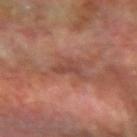The lesion is on the left forearm.
A close-up tile cropped from a whole-body skin photograph, about 15 mm across.
A male patient, roughly 70 years of age.
Automated image analysis of the tile measured an area of roughly 3.5 mm² and an outline eccentricity of about 0.8 (0 = round, 1 = elongated). It also reported border irregularity of about 3.5 on a 0–10 scale, internal color variation of about 1 on a 0–10 scale, and peripheral color asymmetry of about 0.